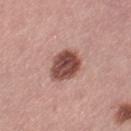<lesion>
<biopsy_status>not biopsied; imaged during a skin examination</biopsy_status>
<image>
  <source>total-body photography crop</source>
  <field_of_view_mm>15</field_of_view_mm>
</image>
<automated_metrics>
  <border_irregularity_0_10>2.0</border_irregularity_0_10>
  <color_variation_0_10>5.5</color_variation_0_10>
  <peripheral_color_asymmetry>2.0</peripheral_color_asymmetry>
  <nevus_likeness_0_100>65</nevus_likeness_0_100>
  <lesion_detection_confidence_0_100>100</lesion_detection_confidence_0_100>
</automated_metrics>
<lesion_size>
  <long_diameter_mm_approx>4.0</long_diameter_mm_approx>
</lesion_size>
<patient>
  <sex>female</sex>
  <age_approx>55</age_approx>
</patient>
<site>left thigh</site>
</lesion>